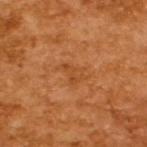biopsy status=catalogued during a skin exam; not biopsied | subject=male, approximately 65 years of age | image source=~15 mm tile from a whole-body skin photo.